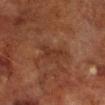<case>
<biopsy_status>not biopsied; imaged during a skin examination</biopsy_status>
<patient>
  <sex>male</sex>
  <age_approx>70</age_approx>
</patient>
<lesion_size>
  <long_diameter_mm_approx>3.5</long_diameter_mm_approx>
</lesion_size>
<lighting>cross-polarized</lighting>
<site>right lower leg</site>
<image>
  <source>total-body photography crop</source>
  <field_of_view_mm>15</field_of_view_mm>
</image>
</case>